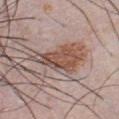Recorded during total-body skin imaging; not selected for excision or biopsy.